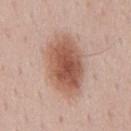Assessment: Recorded during total-body skin imaging; not selected for excision or biopsy. Image and clinical context: Located on the mid back. A region of skin cropped from a whole-body photographic capture, roughly 15 mm wide. A male subject, aged 48–52.